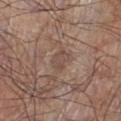Captured during whole-body skin photography for melanoma surveillance; the lesion was not biopsied. On the left lower leg. The subject is a male aged approximately 70. Automated image analysis of the tile measured a footprint of about 4.5 mm², an outline eccentricity of about 0.7 (0 = round, 1 = elongated), and a shape-asymmetry score of about 0.25 (0 = symmetric). The software also gave an average lesion color of about L≈48 a*≈17 b*≈23 (CIELAB), a lesion–skin lightness drop of about 6, and a normalized border contrast of about 5. The analysis additionally found an automated nevus-likeness rating near 0 out of 100 and a detector confidence of about 90 out of 100 that the crop contains a lesion. A 15 mm close-up extracted from a 3D total-body photography capture. Approximately 2.5 mm at its widest.